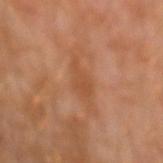follow-up = total-body-photography surveillance lesion; no biopsy | image = total-body-photography crop, ~15 mm field of view | site = the left forearm | patient = male, roughly 30 years of age | size = about 4 mm.